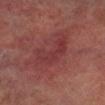Q: Was a biopsy performed?
A: catalogued during a skin exam; not biopsied
Q: What are the patient's age and sex?
A: male, about 75 years old
Q: What kind of image is this?
A: ~15 mm crop, total-body skin-cancer survey
Q: How large is the lesion?
A: ≈5 mm
Q: Illumination type?
A: cross-polarized illumination
Q: What is the anatomic site?
A: the right lower leg
Q: What did automated image analysis measure?
A: a mean CIELAB color near L≈29 a*≈23 b*≈18, a lesion–skin lightness drop of about 5, and a lesion-to-skin contrast of about 5 (normalized; higher = more distinct); border irregularity of about 6 on a 0–10 scale, a within-lesion color-variation index near 3/10, and a peripheral color-asymmetry measure near 1